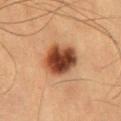follow-up — no biopsy performed (imaged during a skin exam)
automated metrics — a lesion area of about 14 mm² and an outline eccentricity of about 0.5 (0 = round, 1 = elongated); an average lesion color of about L≈39 a*≈22 b*≈30 (CIELAB), a lesion–skin lightness drop of about 18, and a normalized border contrast of about 13.5; a lesion-detection confidence of about 100/100
body site — the abdomen
subject — male, in their mid- to late 50s
image — 15 mm crop, total-body photography
illumination — cross-polarized illumination